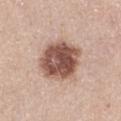Q: Is there a histopathology result?
A: no biopsy performed (imaged during a skin exam)
Q: Who is the patient?
A: female, aged around 45
Q: What did automated image analysis measure?
A: a mean CIELAB color near L≈52 a*≈20 b*≈26 and a lesion–skin lightness drop of about 18; an automated nevus-likeness rating near 55 out of 100 and lesion-presence confidence of about 100/100
Q: Lesion location?
A: the left thigh
Q: Lesion size?
A: ≈6 mm
Q: What kind of image is this?
A: total-body-photography crop, ~15 mm field of view
Q: What lighting was used for the tile?
A: white-light